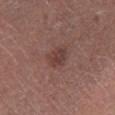From the right lower leg.
A 15 mm crop from a total-body photograph taken for skin-cancer surveillance.
A male patient aged 63–67.
The recorded lesion diameter is about 2.5 mm.